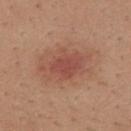| feature | finding |
|---|---|
| patient | male, aged 18 to 22 |
| site | the mid back |
| image source | ~15 mm crop, total-body skin-cancer survey |
| automated metrics | a normalized border contrast of about 6.5; border irregularity of about 4 on a 0–10 scale, internal color variation of about 2 on a 0–10 scale, and peripheral color asymmetry of about 0.5; a classifier nevus-likeness of about 45/100 and a lesion-detection confidence of about 100/100 |
| illumination | white-light illumination |
| diameter | ~5.5 mm (longest diameter) |